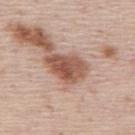Assessment: Imaged during a routine full-body skin examination; the lesion was not biopsied and no histopathology is available. Context: A 15 mm crop from a total-body photograph taken for skin-cancer surveillance. The lesion is on the mid back. A male subject about 45 years old.